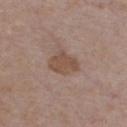About 3.5 mm across.
The subject is a male aged 48 to 52.
This is a white-light tile.
A 15 mm crop from a total-body photograph taken for skin-cancer surveillance.
From the chest.
An algorithmic analysis of the crop reported an outline eccentricity of about 0.7 (0 = round, 1 = elongated) and two-axis asymmetry of about 0.25. The analysis additionally found a lesion color around L≈49 a*≈17 b*≈26 in CIELAB, about 8 CIELAB-L* units darker than the surrounding skin, and a lesion-to-skin contrast of about 7 (normalized; higher = more distinct). The software also gave border irregularity of about 2.5 on a 0–10 scale, a within-lesion color-variation index near 1.5/10, and a peripheral color-asymmetry measure near 0.5.